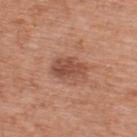{
  "biopsy_status": "not biopsied; imaged during a skin examination",
  "image": {
    "source": "total-body photography crop",
    "field_of_view_mm": 15
  },
  "lighting": "white-light",
  "patient": {
    "sex": "male",
    "age_approx": 70
  },
  "site": "upper back",
  "automated_metrics": {
    "vs_skin_darker_L": 11.0,
    "border_irregularity_0_10": 3.0,
    "color_variation_0_10": 3.5,
    "nevus_likeness_0_100": 45
  }
}